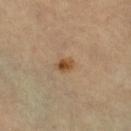{
  "biopsy_status": "not biopsied; imaged during a skin examination",
  "lesion_size": {
    "long_diameter_mm_approx": 2.0
  },
  "automated_metrics": {
    "cielab_L": 50,
    "cielab_a": 19,
    "cielab_b": 37,
    "vs_skin_darker_L": 11.0,
    "color_variation_0_10": 4.0,
    "peripheral_color_asymmetry": 1.5
  },
  "lighting": "cross-polarized",
  "image": {
    "source": "total-body photography crop",
    "field_of_view_mm": 15
  },
  "patient": {
    "sex": "female",
    "age_approx": 60
  },
  "site": "left leg"
}